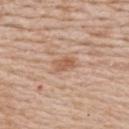biopsy_status: not biopsied; imaged during a skin examination
lighting: white-light
lesion_size:
  long_diameter_mm_approx: 3.0
image:
  source: total-body photography crop
  field_of_view_mm: 15
patient:
  sex: female
  age_approx: 50
site: upper back